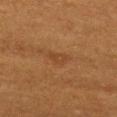Part of a total-body skin-imaging series; this lesion was reviewed on a skin check and was not flagged for biopsy. The tile uses cross-polarized illumination. The lesion is on the chest. A 15 mm crop from a total-body photograph taken for skin-cancer surveillance. Measured at roughly 3 mm in maximum diameter. A female subject in their mid-50s.